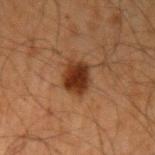| feature | finding |
|---|---|
| workup | imaged on a skin check; not biopsied |
| lighting | cross-polarized illumination |
| subject | male, aged around 65 |
| location | the left upper arm |
| lesion size | ≈3.5 mm |
| image source | ~15 mm crop, total-body skin-cancer survey |